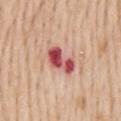The lesion was tiled from a total-body skin photograph and was not biopsied. Located on the mid back. Automated image analysis of the tile measured a footprint of about 8 mm², a shape eccentricity near 0.85, and two-axis asymmetry of about 0.3. And it measured an average lesion color of about L≈53 a*≈34 b*≈25 (CIELAB), a lesion–skin lightness drop of about 18, and a lesion-to-skin contrast of about 11.5 (normalized; higher = more distinct). The software also gave border irregularity of about 3 on a 0–10 scale, a color-variation rating of about 10/10, and peripheral color asymmetry of about 3.5. And it measured a nevus-likeness score of about 0/100. Approximately 4 mm at its widest. A male patient, aged approximately 60. A roughly 15 mm field-of-view crop from a total-body skin photograph.